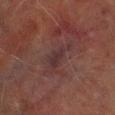biopsy_status: not biopsied; imaged during a skin examination
automated_metrics:
  border_irregularity_0_10: 5.5
  color_variation_0_10: 2.0
  peripheral_color_asymmetry: 0.5
  nevus_likeness_0_100: 0
  lesion_detection_confidence_0_100: 70
lighting: cross-polarized
site: right lower leg
image:
  source: total-body photography crop
  field_of_view_mm: 15
patient:
  sex: male
  age_approx: 70